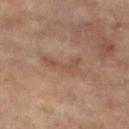Q: Was this lesion biopsied?
A: no biopsy performed (imaged during a skin exam)
Q: What are the patient's age and sex?
A: female, aged around 75
Q: What is the lesion's diameter?
A: about 4.5 mm
Q: What is the imaging modality?
A: ~15 mm crop, total-body skin-cancer survey
Q: Lesion location?
A: the right lower leg